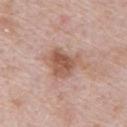Q: Was a biopsy performed?
A: imaged on a skin check; not biopsied
Q: What is the anatomic site?
A: the front of the torso
Q: Lesion size?
A: about 5 mm
Q: What kind of image is this?
A: 15 mm crop, total-body photography
Q: Patient demographics?
A: male, aged 68 to 72
Q: Automated lesion metrics?
A: a mean CIELAB color near L≈57 a*≈20 b*≈28 and a lesion–skin lightness drop of about 11; a color-variation rating of about 4.5/10